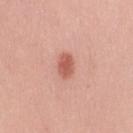This lesion was catalogued during total-body skin photography and was not selected for biopsy.
A 15 mm close-up tile from a total-body photography series done for melanoma screening.
A female subject in their 40s.
Automated image analysis of the tile measured a shape eccentricity near 0.65 and a symmetry-axis asymmetry near 0.15. The software also gave a mean CIELAB color near L≈58 a*≈28 b*≈29 and a lesion–skin lightness drop of about 12. And it measured a lesion-detection confidence of about 100/100.
This is a white-light tile.
Located on the right upper arm.
Approximately 3 mm at its widest.